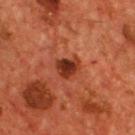  biopsy_status: not biopsied; imaged during a skin examination
  automated_metrics:
    shape_asymmetry: 0.3
    color_variation_0_10: 4.0
    peripheral_color_asymmetry: 1.0
  image:
    source: total-body photography crop
    field_of_view_mm: 15
  patient:
    sex: male
    age_approx: 55
  site: front of the torso